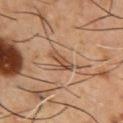follow-up: catalogued during a skin exam; not biopsied
body site: the chest
image: ~15 mm crop, total-body skin-cancer survey
tile lighting: cross-polarized illumination
subject: male, aged 53–57
image-analysis metrics: a shape-asymmetry score of about 0.4 (0 = symmetric); an average lesion color of about L≈48 a*≈20 b*≈31 (CIELAB), a lesion–skin lightness drop of about 10, and a normalized lesion–skin contrast near 7.5
size: ~3.5 mm (longest diameter)